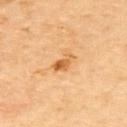Imaged during a routine full-body skin examination; the lesion was not biopsied and no histopathology is available.